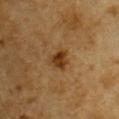Part of a total-body skin-imaging series; this lesion was reviewed on a skin check and was not flagged for biopsy. Approximately 3 mm at its widest. A male patient aged approximately 85 or older. The tile uses cross-polarized illumination. Automated tile analysis of the lesion measured a mean CIELAB color near L≈32 a*≈17 b*≈32 and a lesion–skin lightness drop of about 9. Located on the chest. A 15 mm close-up extracted from a 3D total-body photography capture.